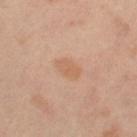Captured during whole-body skin photography for melanoma surveillance; the lesion was not biopsied. The lesion is located on the left thigh. Cropped from a whole-body photographic skin survey; the tile spans about 15 mm. Automated tile analysis of the lesion measured a lesion–skin lightness drop of about 6 and a normalized border contrast of about 5. It also reported a border-irregularity index near 2/10 and internal color variation of about 1.5 on a 0–10 scale. A female subject roughly 50 years of age. The tile uses cross-polarized illumination.